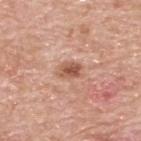notes=catalogued during a skin exam; not biopsied